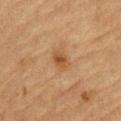| feature | finding |
|---|---|
| notes | total-body-photography surveillance lesion; no biopsy |
| acquisition | total-body-photography crop, ~15 mm field of view |
| anatomic site | the front of the torso |
| lighting | cross-polarized illumination |
| lesion size | about 2.5 mm |
| subject | male, aged around 85 or older |